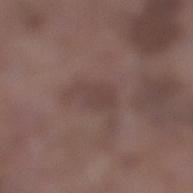Part of a total-body skin-imaging series; this lesion was reviewed on a skin check and was not flagged for biopsy. This is a white-light tile. The subject is a male roughly 75 years of age. A 15 mm close-up extracted from a 3D total-body photography capture. The lesion is located on the right lower leg. Automated tile analysis of the lesion measured a mean CIELAB color near L≈41 a*≈16 b*≈19, about 6 CIELAB-L* units darker than the surrounding skin, and a normalized border contrast of about 5. It also reported a nevus-likeness score of about 0/100. The recorded lesion diameter is about 4 mm.